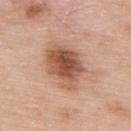Part of a total-body skin-imaging series; this lesion was reviewed on a skin check and was not flagged for biopsy. A male patient about 55 years old. The lesion is located on the upper back. Cropped from a total-body skin-imaging series; the visible field is about 15 mm. The total-body-photography lesion software estimated a lesion area of about 17 mm², a shape eccentricity near 0.75, and two-axis asymmetry of about 0.2. The software also gave an average lesion color of about L≈54 a*≈23 b*≈31 (CIELAB) and a lesion–skin lightness drop of about 14. The analysis additionally found a border-irregularity index near 3/10, a within-lesion color-variation index near 7/10, and radial color variation of about 2. Measured at roughly 6 mm in maximum diameter. Captured under white-light illumination.